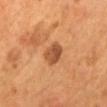workup: imaged on a skin check; not biopsied | acquisition: total-body-photography crop, ~15 mm field of view | body site: the mid back | subject: male, approximately 55 years of age | lighting: cross-polarized illumination.